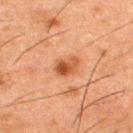No biopsy was performed on this lesion — it was imaged during a full skin examination and was not determined to be concerning.
A male subject, aged approximately 50.
The lesion is located on the back.
An algorithmic analysis of the crop reported an outline eccentricity of about 0.6 (0 = round, 1 = elongated). The software also gave roughly 11 lightness units darker than nearby skin. The software also gave a border-irregularity index near 2.5/10, a within-lesion color-variation index near 6/10, and radial color variation of about 2.5.
Imaged with cross-polarized lighting.
Longest diameter approximately 2.5 mm.
A lesion tile, about 15 mm wide, cut from a 3D total-body photograph.